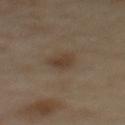The lesion was photographed on a routine skin check and not biopsied; there is no pathology result. Longest diameter approximately 2.5 mm. A female subject, in their 60s. Automated image analysis of the tile measured a footprint of about 4 mm², a shape eccentricity near 0.5, and a shape-asymmetry score of about 0.35 (0 = symmetric). It also reported a border-irregularity index near 3/10, a color-variation rating of about 2/10, and peripheral color asymmetry of about 0.5. The software also gave an automated nevus-likeness rating near 80 out of 100 and a detector confidence of about 100 out of 100 that the crop contains a lesion. A region of skin cropped from a whole-body photographic capture, roughly 15 mm wide. Located on the upper back. Imaged with cross-polarized lighting.